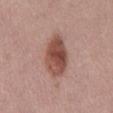Imaged during a routine full-body skin examination; the lesion was not biopsied and no histopathology is available. The patient is a male aged 58–62. The total-body-photography lesion software estimated a lesion area of about 14 mm² and an outline eccentricity of about 0.85 (0 = round, 1 = elongated). The analysis additionally found a mean CIELAB color near L≈49 a*≈23 b*≈25, about 14 CIELAB-L* units darker than the surrounding skin, and a normalized lesion–skin contrast near 10. It also reported a border-irregularity index near 2/10. The software also gave an automated nevus-likeness rating near 90 out of 100. Captured under white-light illumination. The lesion is located on the abdomen. The lesion's longest dimension is about 6 mm. A region of skin cropped from a whole-body photographic capture, roughly 15 mm wide.